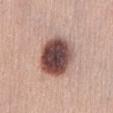The lesion is located on the abdomen.
This image is a 15 mm lesion crop taken from a total-body photograph.
The subject is a female roughly 35 years of age.
Captured under white-light illumination.